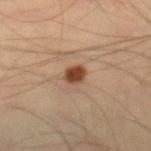Q: Is there a histopathology result?
A: no biopsy performed (imaged during a skin exam)
Q: Patient demographics?
A: male, in their mid-30s
Q: Illumination type?
A: cross-polarized
Q: Lesion location?
A: the right lower leg
Q: What is the imaging modality?
A: total-body-photography crop, ~15 mm field of view
Q: What is the lesion's diameter?
A: about 2.5 mm